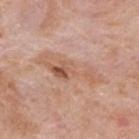This lesion was catalogued during total-body skin photography and was not selected for biopsy. Imaged with white-light lighting. A 15 mm crop from a total-body photograph taken for skin-cancer surveillance. The patient is a male aged approximately 75. The lesion is located on the back.